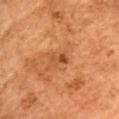Case summary:
* image · ~15 mm tile from a whole-body skin photo
* anatomic site · the head or neck
* patient · female, aged around 65